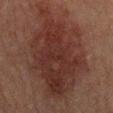A male patient, about 65 years old. The lesion is on the chest. A lesion tile, about 15 mm wide, cut from a 3D total-body photograph.